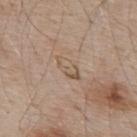Recorded during total-body skin imaging; not selected for excision or biopsy.
A male subject approximately 55 years of age.
The lesion is on the upper back.
A 15 mm crop from a total-body photograph taken for skin-cancer surveillance.
About 4 mm across.